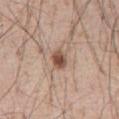Impression: Imaged during a routine full-body skin examination; the lesion was not biopsied and no histopathology is available. Acquisition and patient details: The total-body-photography lesion software estimated a footprint of about 4 mm², an outline eccentricity of about 0.65 (0 = round, 1 = elongated), and two-axis asymmetry of about 0.3. The software also gave a nevus-likeness score of about 95/100 and a detector confidence of about 100 out of 100 that the crop contains a lesion. This is a white-light tile. From the front of the torso. A male patient about 55 years old. This image is a 15 mm lesion crop taken from a total-body photograph.